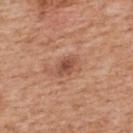follow-up — no biopsy performed (imaged during a skin exam) | location — the upper back | subject — male, aged approximately 60 | TBP lesion metrics — an area of roughly 4 mm², a shape eccentricity near 0.75, and a shape-asymmetry score of about 0.3 (0 = symmetric); a mean CIELAB color near L≈50 a*≈24 b*≈31, a lesion–skin lightness drop of about 10, and a normalized border contrast of about 7.5; a border-irregularity index near 3/10, internal color variation of about 2.5 on a 0–10 scale, and peripheral color asymmetry of about 0.5 | lesion size — about 2.5 mm | image — 15 mm crop, total-body photography.